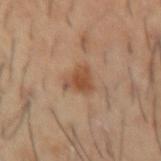workup: no biopsy performed (imaged during a skin exam)
subject: male, aged 53–57
imaging modality: total-body-photography crop, ~15 mm field of view
tile lighting: cross-polarized
anatomic site: the right forearm
size: ≈3.5 mm
image-analysis metrics: a shape-asymmetry score of about 0.45 (0 = symmetric); border irregularity of about 4.5 on a 0–10 scale, internal color variation of about 3 on a 0–10 scale, and a peripheral color-asymmetry measure near 1